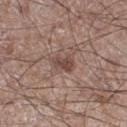Part of a total-body skin-imaging series; this lesion was reviewed on a skin check and was not flagged for biopsy. The lesion is located on the right thigh. The tile uses white-light illumination. The recorded lesion diameter is about 3 mm. The lesion-visualizer software estimated a mean CIELAB color near L≈45 a*≈18 b*≈23, a lesion–skin lightness drop of about 10, and a normalized lesion–skin contrast near 8. The analysis additionally found a classifier nevus-likeness of about 40/100. This image is a 15 mm lesion crop taken from a total-body photograph. A male patient aged 58 to 62.